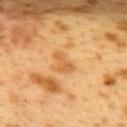Clinical impression:
The lesion was photographed on a routine skin check and not biopsied; there is no pathology result.
Image and clinical context:
The subject is a female aged 38 to 42. From the upper back. A close-up tile cropped from a whole-body skin photograph, about 15 mm across.